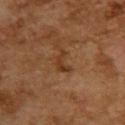The lesion was tiled from a total-body skin photograph and was not biopsied. Automated tile analysis of the lesion measured roughly 7 lightness units darker than nearby skin and a lesion-to-skin contrast of about 7 (normalized; higher = more distinct). The analysis additionally found a color-variation rating of about 0/10 and radial color variation of about 0. The software also gave an automated nevus-likeness rating near 0 out of 100 and a lesion-detection confidence of about 100/100. The lesion is on the upper back. A roughly 15 mm field-of-view crop from a total-body skin photograph. Captured under cross-polarized illumination. A female patient, aged 58–62. Measured at roughly 3 mm in maximum diameter.